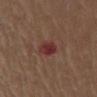| feature | finding |
|---|---|
| biopsy status | catalogued during a skin exam; not biopsied |
| lighting | white-light |
| anatomic site | the chest |
| lesion diameter | ≈2.5 mm |
| patient | male, aged approximately 75 |
| image-analysis metrics | a lesion area of about 5 mm² and a symmetry-axis asymmetry near 0.15; a normalized lesion–skin contrast near 9; a nevus-likeness score of about 0/100 and lesion-presence confidence of about 100/100 |
| acquisition | ~15 mm crop, total-body skin-cancer survey |